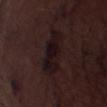follow-up: no biopsy performed (imaged during a skin exam) | anatomic site: the leg | acquisition: total-body-photography crop, ~15 mm field of view | subject: male, in their mid-70s.